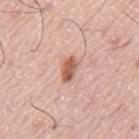workup: imaged on a skin check; not biopsied
anatomic site: the mid back
lesion diameter: ≈3 mm
TBP lesion metrics: a lesion area of about 4 mm² and a symmetry-axis asymmetry near 0.25; a lesion–skin lightness drop of about 14 and a normalized border contrast of about 9.5; internal color variation of about 3 on a 0–10 scale and a peripheral color-asymmetry measure near 1; a nevus-likeness score of about 95/100 and a lesion-detection confidence of about 100/100
patient: male, approximately 75 years of age
imaging modality: ~15 mm tile from a whole-body skin photo